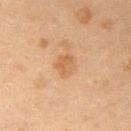Findings:
- tile lighting — cross-polarized
- body site — the right upper arm
- lesion diameter — about 2.5 mm
- imaging modality — ~15 mm tile from a whole-body skin photo
- subject — female, aged 38–42